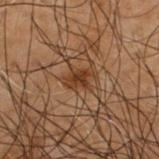Imaged during a routine full-body skin examination; the lesion was not biopsied and no histopathology is available.